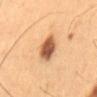Recorded during total-body skin imaging; not selected for excision or biopsy. The lesion is on the lower back. Longest diameter approximately 3.5 mm. The subject is a male aged 58–62. A region of skin cropped from a whole-body photographic capture, roughly 15 mm wide.